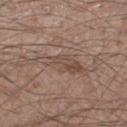Impression:
The lesion was tiled from a total-body skin photograph and was not biopsied.
Image and clinical context:
An algorithmic analysis of the crop reported an outline eccentricity of about 0.95 (0 = round, 1 = elongated) and two-axis asymmetry of about 0.5. It also reported roughly 7 lightness units darker than nearby skin. It also reported a border-irregularity index near 7/10 and a within-lesion color-variation index near 4/10. The analysis additionally found a nevus-likeness score of about 0/100. This image is a 15 mm lesion crop taken from a total-body photograph. A male patient in their 50s. The recorded lesion diameter is about 6 mm. Located on the left thigh. Captured under white-light illumination.